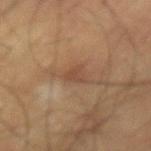Captured under cross-polarized illumination.
Located on the lower back.
The patient is a male in their mid- to late 60s.
About 2.5 mm across.
The lesion-visualizer software estimated a lesion area of about 3 mm², a shape eccentricity near 0.7, and two-axis asymmetry of about 0.4. It also reported border irregularity of about 3.5 on a 0–10 scale, a color-variation rating of about 1/10, and peripheral color asymmetry of about 0.5. The analysis additionally found a nevus-likeness score of about 0/100 and lesion-presence confidence of about 95/100.
Cropped from a whole-body photographic skin survey; the tile spans about 15 mm.